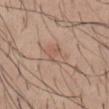workup: imaged on a skin check; not biopsied
lesion diameter: ≈3 mm
subject: male, in their 30s
acquisition: 15 mm crop, total-body photography
body site: the abdomen